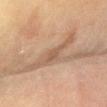Clinical impression:
Imaged during a routine full-body skin examination; the lesion was not biopsied and no histopathology is available.
Background:
A female patient aged approximately 60. Longest diameter approximately 5.5 mm. This is a cross-polarized tile. On the right forearm. A 15 mm crop from a total-body photograph taken for skin-cancer surveillance. Automated image analysis of the tile measured a footprint of about 8 mm², an outline eccentricity of about 0.9 (0 = round, 1 = elongated), and a shape-asymmetry score of about 0.5 (0 = symmetric). It also reported a lesion color around L≈52 a*≈16 b*≈29 in CIELAB. The analysis additionally found a border-irregularity rating of about 7/10 and a color-variation rating of about 2/10. It also reported a nevus-likeness score of about 0/100 and a lesion-detection confidence of about 90/100.